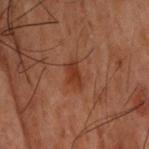Impression:
Captured during whole-body skin photography for melanoma surveillance; the lesion was not biopsied.